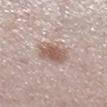Context: Captured under white-light illumination. A 15 mm crop from a total-body photograph taken for skin-cancer surveillance. The lesion is located on the right lower leg. The subject is a male aged approximately 60.